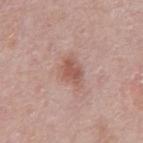No biopsy was performed on this lesion — it was imaged during a full skin examination and was not determined to be concerning. About 4 mm across. A 15 mm crop from a total-body photograph taken for skin-cancer surveillance. Located on the chest. An algorithmic analysis of the crop reported an area of roughly 9 mm² and an eccentricity of roughly 0.75. And it measured border irregularity of about 2.5 on a 0–10 scale. And it measured a classifier nevus-likeness of about 5/100 and lesion-presence confidence of about 100/100. Imaged with white-light lighting. A male subject, aged around 70.